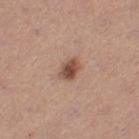No biopsy was performed on this lesion — it was imaged during a full skin examination and was not determined to be concerning. A female patient aged 28 to 32. A region of skin cropped from a whole-body photographic capture, roughly 15 mm wide. Located on the leg. Captured under white-light illumination. The lesion's longest dimension is about 3 mm. Automated image analysis of the tile measured an area of roughly 4.5 mm², an eccentricity of roughly 0.7, and two-axis asymmetry of about 0.2.